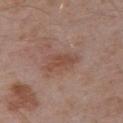The lesion was tiled from a total-body skin photograph and was not biopsied.
The lesion-visualizer software estimated a border-irregularity index near 5/10 and radial color variation of about 0.5. The software also gave a nevus-likeness score of about 5/100 and a detector confidence of about 100 out of 100 that the crop contains a lesion.
The lesion is located on the right upper arm.
A male patient approximately 55 years of age.
Measured at roughly 4 mm in maximum diameter.
Captured under white-light illumination.
A region of skin cropped from a whole-body photographic capture, roughly 15 mm wide.